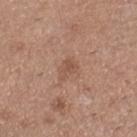Captured during whole-body skin photography for melanoma surveillance; the lesion was not biopsied. An algorithmic analysis of the crop reported a lesion color around L≈52 a*≈20 b*≈28 in CIELAB and roughly 7 lightness units darker than nearby skin. On the right lower leg. A 15 mm close-up tile from a total-body photography series done for melanoma screening. Longest diameter approximately 2.5 mm. This is a white-light tile. The patient is a female aged 28 to 32.